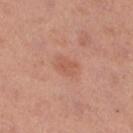Imaged with white-light lighting. A female subject aged approximately 40. The recorded lesion diameter is about 2.5 mm. A close-up tile cropped from a whole-body skin photograph, about 15 mm across. The lesion is located on the right lower leg.